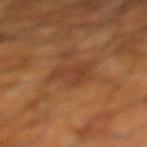biopsy_status: not biopsied; imaged during a skin examination
lighting: cross-polarized
site: left lower leg
lesion_size:
  long_diameter_mm_approx: 4.0
patient:
  sex: male
  age_approx: 60
image:
  source: total-body photography crop
  field_of_view_mm: 15
automated_metrics:
  border_irregularity_0_10: 5.0
  color_variation_0_10: 2.5